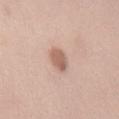notes: catalogued during a skin exam; not biopsied | automated lesion analysis: a mean CIELAB color near L≈60 a*≈20 b*≈27, a lesion–skin lightness drop of about 12, and a lesion-to-skin contrast of about 7.5 (normalized; higher = more distinct) | subject: female, aged 48 to 52 | anatomic site: the mid back | diameter: about 3 mm | image: 15 mm crop, total-body photography.